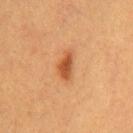<tbp_lesion>
<biopsy_status>not biopsied; imaged during a skin examination</biopsy_status>
<patient>
  <sex>female</sex>
  <age_approx>30</age_approx>
</patient>
<site>chest</site>
<lighting>cross-polarized</lighting>
<lesion_size>
  <long_diameter_mm_approx>3.5</long_diameter_mm_approx>
</lesion_size>
<image>
  <source>total-body photography crop</source>
  <field_of_view_mm>15</field_of_view_mm>
</image>
</tbp_lesion>